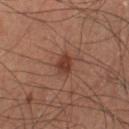<lesion>
<lighting>cross-polarized</lighting>
<automated_metrics>
  <vs_skin_contrast_norm>8.0</vs_skin_contrast_norm>
  <border_irregularity_0_10>2.5</border_irregularity_0_10>
  <color_variation_0_10>2.5</color_variation_0_10>
  <peripheral_color_asymmetry>1.0</peripheral_color_asymmetry>
</automated_metrics>
<site>left lower leg</site>
<image>
  <source>total-body photography crop</source>
  <field_of_view_mm>15</field_of_view_mm>
</image>
<patient>
  <sex>male</sex>
  <age_approx>60</age_approx>
</patient>
<lesion_size>
  <long_diameter_mm_approx>2.5</long_diameter_mm_approx>
</lesion_size>
</lesion>